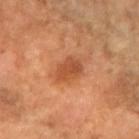| field | value |
|---|---|
| follow-up | no biopsy performed (imaged during a skin exam) |
| image source | 15 mm crop, total-body photography |
| location | the right forearm |
| patient | female, roughly 70 years of age |